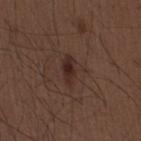Clinical impression: The lesion was photographed on a routine skin check and not biopsied; there is no pathology result. Background: This image is a 15 mm lesion crop taken from a total-body photograph. The lesion is located on the mid back. The subject is a male aged 48 to 52. Captured under white-light illumination. The recorded lesion diameter is about 4 mm.